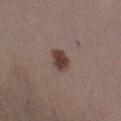Assessment:
No biopsy was performed on this lesion — it was imaged during a full skin examination and was not determined to be concerning.
Clinical summary:
Longest diameter approximately 3 mm. Cropped from a whole-body photographic skin survey; the tile spans about 15 mm. The lesion is on the leg. A female subject aged approximately 30.